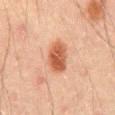Imaged during a routine full-body skin examination; the lesion was not biopsied and no histopathology is available. A 15 mm crop from a total-body photograph taken for skin-cancer surveillance. A male patient roughly 45 years of age. The lesion is located on the front of the torso.